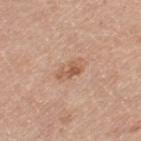This lesion was catalogued during total-body skin photography and was not selected for biopsy.
Approximately 3 mm at its widest.
A close-up tile cropped from a whole-body skin photograph, about 15 mm across.
The lesion-visualizer software estimated internal color variation of about 2.5 on a 0–10 scale and peripheral color asymmetry of about 1. And it measured an automated nevus-likeness rating near 10 out of 100 and lesion-presence confidence of about 100/100.
A female patient, aged 68 to 72.
The lesion is located on the leg.
Captured under white-light illumination.